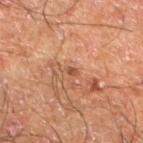Part of a total-body skin-imaging series; this lesion was reviewed on a skin check and was not flagged for biopsy. This is a cross-polarized tile. A male subject aged 58–62. Located on the left lower leg. A close-up tile cropped from a whole-body skin photograph, about 15 mm across. Measured at roughly 1.5 mm in maximum diameter.